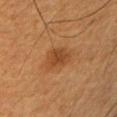Impression: Part of a total-body skin-imaging series; this lesion was reviewed on a skin check and was not flagged for biopsy. Acquisition and patient details: The patient is a male approximately 50 years of age. The recorded lesion diameter is about 3.5 mm. The lesion is on the arm. Captured under cross-polarized illumination. A lesion tile, about 15 mm wide, cut from a 3D total-body photograph. Automated image analysis of the tile measured a footprint of about 7 mm² and a shape eccentricity near 0.55. And it measured a mean CIELAB color near L≈35 a*≈19 b*≈31, about 7 CIELAB-L* units darker than the surrounding skin, and a lesion-to-skin contrast of about 6.5 (normalized; higher = more distinct).